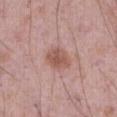biopsy_status: not biopsied; imaged during a skin examination
lesion_size:
  long_diameter_mm_approx: 3.5
patient:
  sex: male
  age_approx: 70
lighting: white-light
site: abdomen
image:
  source: total-body photography crop
  field_of_view_mm: 15